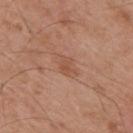Case summary:
• notes — no biopsy performed (imaged during a skin exam)
• subject — male, in their mid-50s
• site — the back
• acquisition — ~15 mm tile from a whole-body skin photo